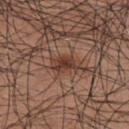Clinical impression:
The lesion was photographed on a routine skin check and not biopsied; there is no pathology result.
Background:
Located on the right upper arm. The tile uses white-light illumination. The recorded lesion diameter is about 3.5 mm. A male patient aged approximately 35. A lesion tile, about 15 mm wide, cut from a 3D total-body photograph.